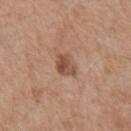Clinical impression: Captured during whole-body skin photography for melanoma surveillance; the lesion was not biopsied. Image and clinical context: A male subject aged around 65. Located on the chest. A roughly 15 mm field-of-view crop from a total-body skin photograph. About 3 mm across.